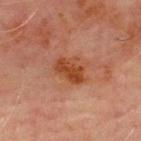Case summary:
* biopsy status — no biopsy performed (imaged during a skin exam)